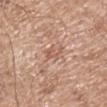Recorded during total-body skin imaging; not selected for excision or biopsy. A male subject, aged 68 to 72. Cropped from a total-body skin-imaging series; the visible field is about 15 mm. Imaged with white-light lighting. The lesion is located on the right upper arm.